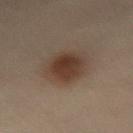The lesion was tiled from a total-body skin photograph and was not biopsied. A 15 mm crop from a total-body photograph taken for skin-cancer surveillance. From the lower back. A female patient about 30 years old.